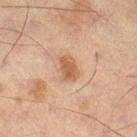Assessment:
No biopsy was performed on this lesion — it was imaged during a full skin examination and was not determined to be concerning.